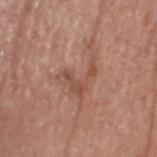Q: Was a biopsy performed?
A: catalogued during a skin exam; not biopsied
Q: Who is the patient?
A: male, aged approximately 60
Q: What is the imaging modality?
A: total-body-photography crop, ~15 mm field of view
Q: Lesion location?
A: the head or neck